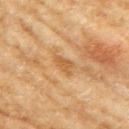Findings:
- follow-up · catalogued during a skin exam; not biopsied
- lesion diameter · about 2.5 mm
- location · the right upper arm
- imaging modality · ~15 mm crop, total-body skin-cancer survey
- automated metrics · a lesion area of about 3.5 mm², an eccentricity of roughly 0.75, and a shape-asymmetry score of about 0.35 (0 = symmetric)
- lighting · cross-polarized
- subject · female, aged around 60